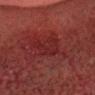<lesion>
  <biopsy_status>not biopsied; imaged during a skin examination</biopsy_status>
</lesion>